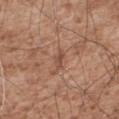A male subject, in their mid-50s. A roughly 15 mm field-of-view crop from a total-body skin photograph. Imaged with white-light lighting. From the upper back.